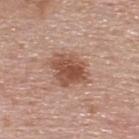Q: How large is the lesion?
A: ~5 mm (longest diameter)
Q: What kind of image is this?
A: ~15 mm crop, total-body skin-cancer survey
Q: Who is the patient?
A: male, aged 53–57
Q: Lesion location?
A: the upper back